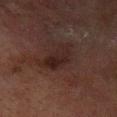Impression:
Imaged during a routine full-body skin examination; the lesion was not biopsied and no histopathology is available.
Image and clinical context:
About 4.5 mm across. Automated image analysis of the tile measured an average lesion color of about L≈18 a*≈14 b*≈15 (CIELAB), a lesion–skin lightness drop of about 6, and a normalized lesion–skin contrast near 7.5. And it measured a border-irregularity index near 3.5/10, a color-variation rating of about 4/10, and peripheral color asymmetry of about 1.5. The software also gave a nevus-likeness score of about 25/100. Cropped from a total-body skin-imaging series; the visible field is about 15 mm. The subject is a male aged approximately 70. This is a cross-polarized tile. The lesion is on the left lower leg.